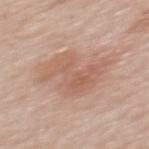  automated_metrics:
    cielab_L: 60
    cielab_a: 20
    cielab_b: 29
    vs_skin_darker_L: 8.0
    vs_skin_contrast_norm: 6.0
    lesion_detection_confidence_0_100: 100
  lesion_size:
    long_diameter_mm_approx: 6.5
  site: upper back
  patient:
    sex: female
    age_approx: 60
  image:
    source: total-body photography crop
    field_of_view_mm: 15
  lighting: white-light